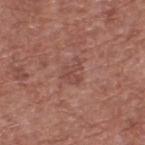notes: catalogued during a skin exam; not biopsied